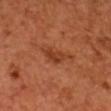biopsy status: imaged on a skin check; not biopsied
patient: male, aged approximately 50
image: total-body-photography crop, ~15 mm field of view
location: the head or neck